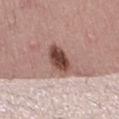The lesion was tiled from a total-body skin photograph and was not biopsied. The patient is a female aged 48–52. The lesion is located on the left thigh. This is a white-light tile. Measured at roughly 4 mm in maximum diameter. Cropped from a total-body skin-imaging series; the visible field is about 15 mm.